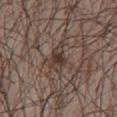Part of a total-body skin-imaging series; this lesion was reviewed on a skin check and was not flagged for biopsy. An algorithmic analysis of the crop reported a lesion area of about 6.5 mm², an outline eccentricity of about 0.8 (0 = round, 1 = elongated), and two-axis asymmetry of about 0.3. The analysis additionally found an average lesion color of about L≈37 a*≈14 b*≈22 (CIELAB). A male patient roughly 65 years of age. Cropped from a total-body skin-imaging series; the visible field is about 15 mm. This is a white-light tile. Located on the chest. Longest diameter approximately 3.5 mm.